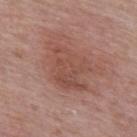Imaged during a routine full-body skin examination; the lesion was not biopsied and no histopathology is available. A lesion tile, about 15 mm wide, cut from a 3D total-body photograph. A female patient, aged approximately 60. On the upper back.